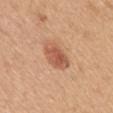Impression: Imaged during a routine full-body skin examination; the lesion was not biopsied and no histopathology is available. Clinical summary: On the mid back. The lesion-visualizer software estimated a lesion color around L≈57 a*≈24 b*≈34 in CIELAB and a lesion–skin lightness drop of about 11. The software also gave a border-irregularity index near 1.5/10, internal color variation of about 4 on a 0–10 scale, and peripheral color asymmetry of about 1.5. Imaged with white-light lighting. A 15 mm close-up extracted from a 3D total-body photography capture. Measured at roughly 4.5 mm in maximum diameter. A female subject roughly 55 years of age.